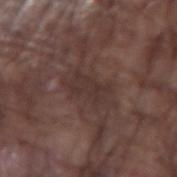Clinical impression:
Recorded during total-body skin imaging; not selected for excision or biopsy.
Image and clinical context:
A male subject, roughly 75 years of age. From the arm. Cropped from a whole-body photographic skin survey; the tile spans about 15 mm. Imaged with white-light lighting. About 4 mm across.